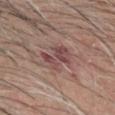• notes: total-body-photography surveillance lesion; no biopsy
• body site: the head or neck
• lesion size: ~4.5 mm (longest diameter)
• patient: male, in their mid- to late 60s
• image source: ~15 mm tile from a whole-body skin photo